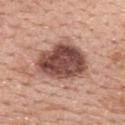Assessment: Recorded during total-body skin imaging; not selected for excision or biopsy. Background: The lesion's longest dimension is about 7 mm. Captured under white-light illumination. Cropped from a whole-body photographic skin survey; the tile spans about 15 mm. A female subject, in their 60s. On the back.